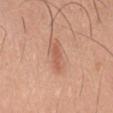Clinical impression:
The lesion was photographed on a routine skin check and not biopsied; there is no pathology result.
Clinical summary:
Automated tile analysis of the lesion measured an area of roughly 7 mm², an outline eccentricity of about 0.9 (0 = round, 1 = elongated), and two-axis asymmetry of about 0.25. The analysis additionally found an automated nevus-likeness rating near 65 out of 100 and a detector confidence of about 100 out of 100 that the crop contains a lesion. The lesion is located on the front of the torso. Cropped from a whole-body photographic skin survey; the tile spans about 15 mm. Measured at roughly 4.5 mm in maximum diameter. This is a white-light tile. A male patient approximately 45 years of age.